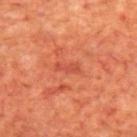A close-up tile cropped from a whole-body skin photograph, about 15 mm across.
The patient is a male roughly 70 years of age.
Longest diameter approximately 2.5 mm.
The lesion is on the upper back.
This is a cross-polarized tile.